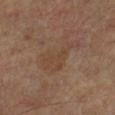location: the right lower leg | patient: male, in their mid- to late 60s | image: 15 mm crop, total-body photography | automated lesion analysis: a lesion area of about 11 mm², an eccentricity of roughly 0.9, and a shape-asymmetry score of about 0.55 (0 = symmetric); a border-irregularity index near 6.5/10, internal color variation of about 1.5 on a 0–10 scale, and radial color variation of about 0.5; a classifier nevus-likeness of about 0/100 and lesion-presence confidence of about 100/100 | lighting: cross-polarized illumination.